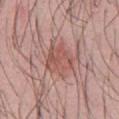No biopsy was performed on this lesion — it was imaged during a full skin examination and was not determined to be concerning.
The lesion is on the chest.
Imaged with white-light lighting.
A male subject, aged 28–32.
Measured at roughly 4 mm in maximum diameter.
Cropped from a total-body skin-imaging series; the visible field is about 15 mm.